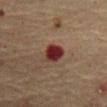Impression:
Recorded during total-body skin imaging; not selected for excision or biopsy.
Clinical summary:
About 2.5 mm across. From the abdomen. The patient is a male aged around 65. A 15 mm close-up extracted from a 3D total-body photography capture.